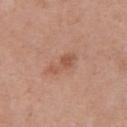Impression: Imaged during a routine full-body skin examination; the lesion was not biopsied and no histopathology is available. Image and clinical context: About 4 mm across. A close-up tile cropped from a whole-body skin photograph, about 15 mm across. From the chest. Captured under white-light illumination. The subject is a male approximately 70 years of age.